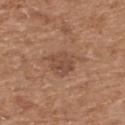Q: Was a biopsy performed?
A: catalogued during a skin exam; not biopsied
Q: What did automated image analysis measure?
A: a border-irregularity rating of about 4/10 and a color-variation rating of about 2.5/10
Q: How was the tile lit?
A: white-light
Q: How large is the lesion?
A: ≈5 mm
Q: Patient demographics?
A: male, about 70 years old
Q: How was this image acquired?
A: total-body-photography crop, ~15 mm field of view
Q: Lesion location?
A: the upper back